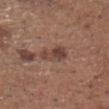Notes:
- notes — no biopsy performed (imaged during a skin exam)
- lesion size — ≈3.5 mm
- patient — male, aged 73–77
- lighting — white-light illumination
- acquisition — ~15 mm tile from a whole-body skin photo
- image-analysis metrics — a lesion area of about 5.5 mm², a shape eccentricity near 0.85, and a symmetry-axis asymmetry near 0.35; an average lesion color of about L≈42 a*≈18 b*≈24 (CIELAB) and a lesion–skin lightness drop of about 10; an automated nevus-likeness rating near 15 out of 100 and a detector confidence of about 100 out of 100 that the crop contains a lesion
- body site — the head or neck